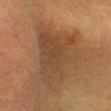| field | value |
|---|---|
| notes | total-body-photography surveillance lesion; no biopsy |
| patient | female, aged 53–57 |
| anatomic site | the head or neck |
| tile lighting | cross-polarized |
| acquisition | ~15 mm crop, total-body skin-cancer survey |